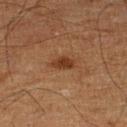Context:
A 15 mm close-up tile from a total-body photography series done for melanoma screening. The lesion's longest dimension is about 3 mm. Imaged with cross-polarized lighting. Automated tile analysis of the lesion measured a footprint of about 4.5 mm². It also reported a classifier nevus-likeness of about 80/100 and a detector confidence of about 100 out of 100 that the crop contains a lesion. From the left lower leg. A male subject aged 73–77.